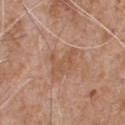Assessment:
The lesion was photographed on a routine skin check and not biopsied; there is no pathology result.
Clinical summary:
Automated tile analysis of the lesion measured an area of roughly 5 mm², an outline eccentricity of about 0.85 (0 = round, 1 = elongated), and two-axis asymmetry of about 0.5. It also reported a mean CIELAB color near L≈54 a*≈21 b*≈32, a lesion–skin lightness drop of about 6, and a normalized border contrast of about 5. The software also gave a nevus-likeness score of about 0/100 and lesion-presence confidence of about 100/100. From the chest. A male patient, aged approximately 65. Imaged with white-light lighting. Longest diameter approximately 3.5 mm. This image is a 15 mm lesion crop taken from a total-body photograph.